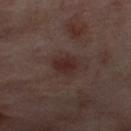Background:
Cropped from a total-body skin-imaging series; the visible field is about 15 mm. The tile uses cross-polarized illumination. The subject is a female roughly 55 years of age. The lesion is located on the right thigh.